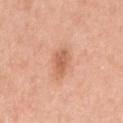Assessment:
Recorded during total-body skin imaging; not selected for excision or biopsy.
Context:
This image is a 15 mm lesion crop taken from a total-body photograph. From the right upper arm. A male subject, roughly 60 years of age.